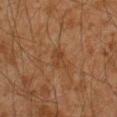No biopsy was performed on this lesion — it was imaged during a full skin examination and was not determined to be concerning. The lesion's longest dimension is about 3 mm. A male patient, in their mid- to late 40s. A 15 mm close-up tile from a total-body photography series done for melanoma screening. Imaged with cross-polarized lighting. The lesion-visualizer software estimated a footprint of about 3.5 mm², a shape eccentricity near 0.8, and a shape-asymmetry score of about 0.25 (0 = symmetric). The analysis additionally found roughly 5 lightness units darker than nearby skin and a normalized lesion–skin contrast near 5.5. It also reported a within-lesion color-variation index near 2/10 and peripheral color asymmetry of about 0.5. It also reported a nevus-likeness score of about 0/100 and lesion-presence confidence of about 100/100. On the right forearm.